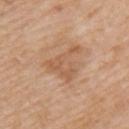Background: The tile uses white-light illumination. Located on the arm. A region of skin cropped from a whole-body photographic capture, roughly 15 mm wide. About 5 mm across. The patient is a male aged approximately 75.